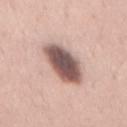location: the mid back
illumination: white-light
patient: female, approximately 35 years of age
image: 15 mm crop, total-body photography
size: ~6 mm (longest diameter)